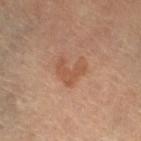Recorded during total-body skin imaging; not selected for excision or biopsy.
The patient is a female aged 63–67.
On the left lower leg.
A 15 mm crop from a total-body photograph taken for skin-cancer surveillance.
The tile uses cross-polarized illumination.